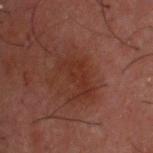| key | value |
|---|---|
| notes | imaged on a skin check; not biopsied |
| subject | male, aged approximately 50 |
| location | the head or neck |
| automated metrics | a nevus-likeness score of about 0/100 |
| acquisition | 15 mm crop, total-body photography |
| tile lighting | cross-polarized illumination |